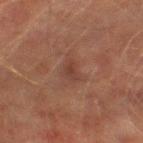Clinical impression: No biopsy was performed on this lesion — it was imaged during a full skin examination and was not determined to be concerning. Acquisition and patient details: The tile uses cross-polarized illumination. A 15 mm close-up tile from a total-body photography series done for melanoma screening. The patient is a male approximately 75 years of age. Automated tile analysis of the lesion measured an area of roughly 4 mm² and a shape-asymmetry score of about 0.35 (0 = symmetric). The software also gave a lesion-to-skin contrast of about 5.5 (normalized; higher = more distinct). The analysis additionally found a nevus-likeness score of about 0/100 and a lesion-detection confidence of about 100/100. From the right thigh. Approximately 3 mm at its widest.